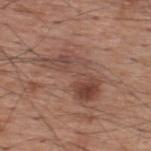Q: Where on the body is the lesion?
A: the upper back
Q: Who is the patient?
A: male, roughly 60 years of age
Q: What is the lesion's diameter?
A: about 7.5 mm
Q: Illumination type?
A: white-light illumination
Q: How was this image acquired?
A: total-body-photography crop, ~15 mm field of view